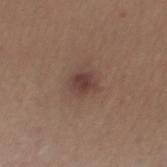patient: male, aged 33 to 37 | image: ~15 mm crop, total-body skin-cancer survey | lesion diameter: ~2.5 mm (longest diameter) | TBP lesion metrics: a footprint of about 5.5 mm², an eccentricity of roughly 0.35, and a shape-asymmetry score of about 0.25 (0 = symmetric); roughly 9 lightness units darker than nearby skin and a normalized lesion–skin contrast near 8; border irregularity of about 2 on a 0–10 scale, a within-lesion color-variation index near 3/10, and a peripheral color-asymmetry measure near 1; a classifier nevus-likeness of about 80/100 and a detector confidence of about 100 out of 100 that the crop contains a lesion | lighting: white-light | location: the mid back.